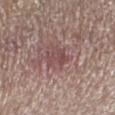No biopsy was performed on this lesion — it was imaged during a full skin examination and was not determined to be concerning.
Automated tile analysis of the lesion measured an area of roughly 4.5 mm², an eccentricity of roughly 0.7, and a shape-asymmetry score of about 0.4 (0 = symmetric). It also reported a mean CIELAB color near L≈47 a*≈21 b*≈18.
A male subject roughly 65 years of age.
The tile uses white-light illumination.
Located on the left lower leg.
Measured at roughly 3 mm in maximum diameter.
Cropped from a whole-body photographic skin survey; the tile spans about 15 mm.